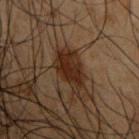<tbp_lesion>
  <biopsy_status>not biopsied; imaged during a skin examination</biopsy_status>
  <image>
    <source>total-body photography crop</source>
    <field_of_view_mm>15</field_of_view_mm>
  </image>
  <site>left upper arm</site>
  <lighting>cross-polarized</lighting>
  <automated_metrics>
    <vs_skin_darker_L>8.0</vs_skin_darker_L>
    <vs_skin_contrast_norm>10.5</vs_skin_contrast_norm>
    <color_variation_0_10>3.0</color_variation_0_10>
    <peripheral_color_asymmetry>1.0</peripheral_color_asymmetry>
    <nevus_likeness_0_100>95</nevus_likeness_0_100>
  </automated_metrics>
  <lesion_size>
    <long_diameter_mm_approx>5.0</long_diameter_mm_approx>
  </lesion_size>
  <patient>
    <sex>male</sex>
    <age_approx>50</age_approx>
  </patient>
</tbp_lesion>